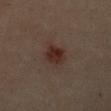Assessment: Part of a total-body skin-imaging series; this lesion was reviewed on a skin check and was not flagged for biopsy. Clinical summary: Captured under cross-polarized illumination. Located on the chest. A female patient, roughly 35 years of age. A roughly 15 mm field-of-view crop from a total-body skin photograph.